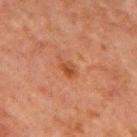The lesion was photographed on a routine skin check and not biopsied; there is no pathology result. The lesion-visualizer software estimated a lesion color around L≈39 a*≈24 b*≈32 in CIELAB, a lesion–skin lightness drop of about 7, and a lesion-to-skin contrast of about 7 (normalized; higher = more distinct). It also reported border irregularity of about 2.5 on a 0–10 scale, internal color variation of about 2.5 on a 0–10 scale, and peripheral color asymmetry of about 1. The software also gave an automated nevus-likeness rating near 55 out of 100. Approximately 2.5 mm at its widest. From the chest. Captured under cross-polarized illumination. A male subject in their mid- to late 60s. A close-up tile cropped from a whole-body skin photograph, about 15 mm across.